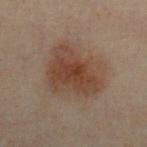Captured during whole-body skin photography for melanoma surveillance; the lesion was not biopsied. A 15 mm crop from a total-body photograph taken for skin-cancer surveillance. A male patient, about 50 years old. This is a cross-polarized tile. The lesion is on the chest. Automated tile analysis of the lesion measured a footprint of about 29 mm², an outline eccentricity of about 0.45 (0 = round, 1 = elongated), and a shape-asymmetry score of about 0.25 (0 = symmetric). The analysis additionally found a border-irregularity index near 2.5/10, a color-variation rating of about 4.5/10, and peripheral color asymmetry of about 1.5. And it measured an automated nevus-likeness rating near 80 out of 100.